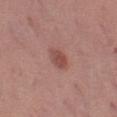Q: Is there a histopathology result?
A: total-body-photography surveillance lesion; no biopsy
Q: What is the anatomic site?
A: the left thigh
Q: How was the tile lit?
A: white-light illumination
Q: What kind of image is this?
A: total-body-photography crop, ~15 mm field of view
Q: What are the patient's age and sex?
A: female, in their 40s
Q: How large is the lesion?
A: ≈3 mm